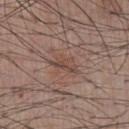From the front of the torso.
Captured under white-light illumination.
Approximately 4 mm at its widest.
A male patient aged 53–57.
Cropped from a whole-body photographic skin survey; the tile spans about 15 mm.